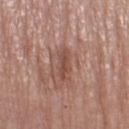* workup — no biopsy performed (imaged during a skin exam)
* site — the right thigh
* image — ~15 mm tile from a whole-body skin photo
* subject — female, in their mid- to late 60s